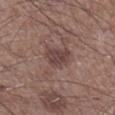Notes:
• biopsy status — total-body-photography surveillance lesion; no biopsy
• subject — male, aged 58–62
• location — the right lower leg
• acquisition — 15 mm crop, total-body photography